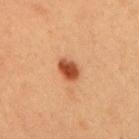Assessment: Imaged during a routine full-body skin examination; the lesion was not biopsied and no histopathology is available. Background: The patient is a female approximately 40 years of age. On the mid back. A lesion tile, about 15 mm wide, cut from a 3D total-body photograph.